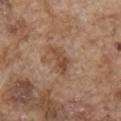The lesion was tiled from a total-body skin photograph and was not biopsied. A close-up tile cropped from a whole-body skin photograph, about 15 mm across. Longest diameter approximately 4 mm. Imaged with white-light lighting. The lesion is on the right upper arm. A male patient approximately 75 years of age. An algorithmic analysis of the crop reported an automated nevus-likeness rating near 15 out of 100.